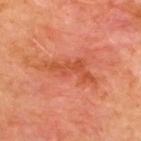Imaged during a routine full-body skin examination; the lesion was not biopsied and no histopathology is available.
Located on the chest.
The subject is a male in their mid- to late 40s.
A close-up tile cropped from a whole-body skin photograph, about 15 mm across.
Measured at roughly 6.5 mm in maximum diameter.